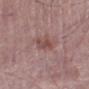{
  "patient": {
    "sex": "male",
    "age_approx": 65
  },
  "lighting": "white-light",
  "lesion_size": {
    "long_diameter_mm_approx": 3.0
  },
  "image": {
    "source": "total-body photography crop",
    "field_of_view_mm": 15
  },
  "automated_metrics": {
    "eccentricity": 0.75,
    "shape_asymmetry": 0.3,
    "border_irregularity_0_10": 3.0,
    "color_variation_0_10": 2.5,
    "peripheral_color_asymmetry": 1.0,
    "lesion_detection_confidence_0_100": 100
  },
  "site": "leg"
}